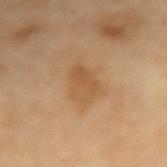Q: Is there a histopathology result?
A: total-body-photography surveillance lesion; no biopsy
Q: How was the tile lit?
A: cross-polarized illumination
Q: Lesion size?
A: ~4 mm (longest diameter)
Q: Lesion location?
A: the arm
Q: Who is the patient?
A: female, approximately 70 years of age
Q: What kind of image is this?
A: 15 mm crop, total-body photography
Q: Automated lesion metrics?
A: an average lesion color of about L≈56 a*≈19 b*≈39 (CIELAB); a detector confidence of about 100 out of 100 that the crop contains a lesion